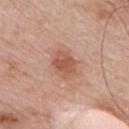Imaged during a routine full-body skin examination; the lesion was not biopsied and no histopathology is available. A male patient, aged 53–57. Cropped from a whole-body photographic skin survey; the tile spans about 15 mm. The tile uses white-light illumination. From the front of the torso.